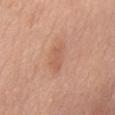Recorded during total-body skin imaging; not selected for excision or biopsy. A female patient roughly 60 years of age. A 15 mm close-up tile from a total-body photography series done for melanoma screening. Automated image analysis of the tile measured a border-irregularity index near 3/10, a within-lesion color-variation index near 1.5/10, and a peripheral color-asymmetry measure near 0.5. From the abdomen. This is a white-light tile.